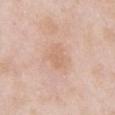The lesion was tiled from a total-body skin photograph and was not biopsied. A male patient in their mid- to late 50s. The lesion is on the front of the torso. A 15 mm close-up extracted from a 3D total-body photography capture.